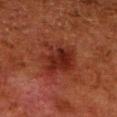Clinical impression:
Part of a total-body skin-imaging series; this lesion was reviewed on a skin check and was not flagged for biopsy.